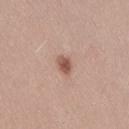{
  "site": "lower back",
  "image": {
    "source": "total-body photography crop",
    "field_of_view_mm": 15
  },
  "patient": {
    "sex": "female",
    "age_approx": 25
  },
  "lighting": "white-light"
}